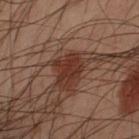No biopsy was performed on this lesion — it was imaged during a full skin examination and was not determined to be concerning.
Longest diameter approximately 3 mm.
A region of skin cropped from a whole-body photographic capture, roughly 15 mm wide.
A male patient, aged 48–52.
The lesion is located on the left forearm.
Imaged with cross-polarized lighting.
The total-body-photography lesion software estimated an average lesion color of about L≈25 a*≈19 b*≈22 (CIELAB) and a normalized lesion–skin contrast near 8.5. The software also gave a border-irregularity rating of about 3.5/10, a color-variation rating of about 1.5/10, and radial color variation of about 0.5. It also reported a lesion-detection confidence of about 100/100.